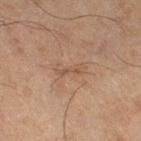Image and clinical context:
Located on the right thigh. A roughly 15 mm field-of-view crop from a total-body skin photograph. Automated image analysis of the tile measured a lesion area of about 3.5 mm², an outline eccentricity of about 0.95 (0 = round, 1 = elongated), and two-axis asymmetry of about 0.45. And it measured an average lesion color of about L≈42 a*≈15 b*≈25 (CIELAB), about 5 CIELAB-L* units darker than the surrounding skin, and a normalized border contrast of about 4.5. About 3.5 mm across. This is a cross-polarized tile. A male subject, aged 63–67.